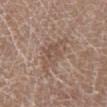Impression: Recorded during total-body skin imaging; not selected for excision or biopsy. Context: The recorded lesion diameter is about 5 mm. Imaged with white-light lighting. A roughly 15 mm field-of-view crop from a total-body skin photograph. The lesion is on the right lower leg. A female patient roughly 80 years of age.